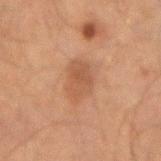Case summary:
– workup — imaged on a skin check; not biopsied
– anatomic site — the arm
– acquisition — ~15 mm crop, total-body skin-cancer survey
– subject — male, aged approximately 50